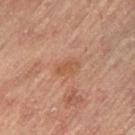The lesion was photographed on a routine skin check and not biopsied; there is no pathology result.
The lesion is on the right leg.
A close-up tile cropped from a whole-body skin photograph, about 15 mm across.
A female patient aged around 80.